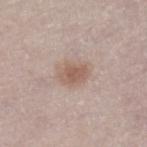| key | value |
|---|---|
| notes | catalogued during a skin exam; not biopsied |
| image | total-body-photography crop, ~15 mm field of view |
| anatomic site | the right lower leg |
| patient | female, aged 58 to 62 |
| illumination | white-light illumination |